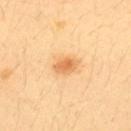Assessment: This lesion was catalogued during total-body skin photography and was not selected for biopsy. Image and clinical context: The tile uses cross-polarized illumination. The lesion's longest dimension is about 3 mm. The subject is a female approximately 35 years of age. A close-up tile cropped from a whole-body skin photograph, about 15 mm across. On the upper back.